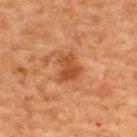Notes:
- notes — catalogued during a skin exam; not biopsied
- subject — male, approximately 65 years of age
- image source — ~15 mm crop, total-body skin-cancer survey
- illumination — cross-polarized illumination
- site — the upper back
- lesion diameter — ~3.5 mm (longest diameter)